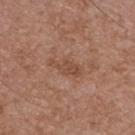Captured during whole-body skin photography for melanoma surveillance; the lesion was not biopsied. A 15 mm close-up extracted from a 3D total-body photography capture. A male patient aged 53 to 57. The recorded lesion diameter is about 4 mm. Located on the front of the torso. Automated tile analysis of the lesion measured a footprint of about 5 mm², an outline eccentricity of about 0.9 (0 = round, 1 = elongated), and a symmetry-axis asymmetry near 0.4. The analysis additionally found a mean CIELAB color near L≈48 a*≈21 b*≈29, a lesion–skin lightness drop of about 7, and a normalized lesion–skin contrast near 5.5. Imaged with white-light lighting.